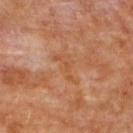biopsy_status: not biopsied; imaged during a skin examination
patient:
  sex: female
  age_approx: 50
site: back
lighting: cross-polarized
automated_metrics:
  area_mm2_approx: 5.0
  shape_asymmetry: 0.55
lesion_size:
  long_diameter_mm_approx: 4.5
image:
  source: total-body photography crop
  field_of_view_mm: 15